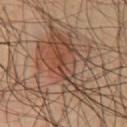{
  "biopsy_status": "not biopsied; imaged during a skin examination",
  "patient": {
    "sex": "male",
    "age_approx": 65
  },
  "site": "front of the torso",
  "image": {
    "source": "total-body photography crop",
    "field_of_view_mm": 15
  },
  "automated_metrics": {
    "cielab_L": 48,
    "cielab_a": 18,
    "cielab_b": 28,
    "vs_skin_darker_L": 8.0,
    "border_irregularity_0_10": 8.5,
    "peripheral_color_asymmetry": 3.0
  },
  "lighting": "cross-polarized"
}